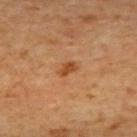Cropped from a whole-body photographic skin survey; the tile spans about 15 mm. A patient aged 68–72. On the upper back.